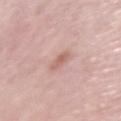<case>
  <biopsy_status>not biopsied; imaged during a skin examination</biopsy_status>
  <lesion_size>
    <long_diameter_mm_approx>2.5</long_diameter_mm_approx>
  </lesion_size>
  <image>
    <source>total-body photography crop</source>
    <field_of_view_mm>15</field_of_view_mm>
  </image>
  <patient>
    <sex>female</sex>
    <age_approx>65</age_approx>
  </patient>
  <lighting>white-light</lighting>
  <site>left upper arm</site>
</case>